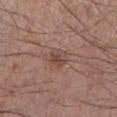Case summary:
* follow-up: total-body-photography surveillance lesion; no biopsy
* lesion size: about 2.5 mm
* anatomic site: the left lower leg
* patient: male, about 65 years old
* acquisition: ~15 mm tile from a whole-body skin photo
* illumination: white-light